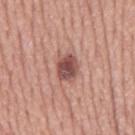| field | value |
|---|---|
| notes | catalogued during a skin exam; not biopsied |
| imaging modality | total-body-photography crop, ~15 mm field of view |
| patient | male, aged 73–77 |
| lesion diameter | ≈3.5 mm |
| body site | the back |
| automated lesion analysis | roughly 15 lightness units darker than nearby skin and a lesion-to-skin contrast of about 10 (normalized; higher = more distinct); lesion-presence confidence of about 100/100 |
| illumination | white-light illumination |